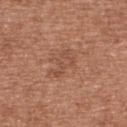| feature | finding |
|---|---|
| workup | imaged on a skin check; not biopsied |
| subject | female, aged 38–42 |
| acquisition | ~15 mm tile from a whole-body skin photo |
| site | the upper back |